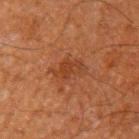follow-up — total-body-photography surveillance lesion; no biopsy
site — the left upper arm
imaging modality — total-body-photography crop, ~15 mm field of view
automated lesion analysis — an area of roughly 6 mm², an eccentricity of roughly 0.75, and a shape-asymmetry score of about 0.35 (0 = symmetric); a lesion color around L≈31 a*≈21 b*≈29 in CIELAB, roughly 6 lightness units darker than nearby skin, and a normalized lesion–skin contrast near 6; a nevus-likeness score of about 0/100 and lesion-presence confidence of about 100/100
subject — male, aged 58–62
diameter — ~3.5 mm (longest diameter)